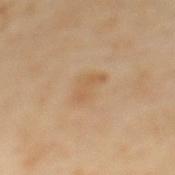The lesion was photographed on a routine skin check and not biopsied; there is no pathology result. A male patient aged 63–67. This is a cross-polarized tile. On the back. A region of skin cropped from a whole-body photographic capture, roughly 15 mm wide. The recorded lesion diameter is about 3.5 mm.